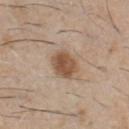workup: imaged on a skin check; not biopsied
acquisition: total-body-photography crop, ~15 mm field of view
subject: male, about 60 years old
body site: the chest